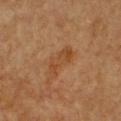No biopsy was performed on this lesion — it was imaged during a full skin examination and was not determined to be concerning.
A female subject aged around 40.
The lesion is located on the chest.
The total-body-photography lesion software estimated an area of roughly 6.5 mm² and a shape eccentricity near 0.9. The software also gave a mean CIELAB color near L≈39 a*≈19 b*≈32, a lesion–skin lightness drop of about 6, and a lesion-to-skin contrast of about 6 (normalized; higher = more distinct). It also reported internal color variation of about 3 on a 0–10 scale and peripheral color asymmetry of about 1.
Measured at roughly 4.5 mm in maximum diameter.
A lesion tile, about 15 mm wide, cut from a 3D total-body photograph.
Imaged with cross-polarized lighting.